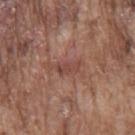The lesion was tiled from a total-body skin photograph and was not biopsied. A close-up tile cropped from a whole-body skin photograph, about 15 mm across. Automated image analysis of the tile measured an area of roughly 4 mm², a shape eccentricity near 0.9, and a symmetry-axis asymmetry near 0.5. The software also gave a lesion-to-skin contrast of about 5.5 (normalized; higher = more distinct). This is a white-light tile. A male patient, about 75 years old. The lesion's longest dimension is about 3.5 mm. The lesion is on the mid back.